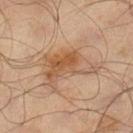Captured during whole-body skin photography for melanoma surveillance; the lesion was not biopsied. A roughly 15 mm field-of-view crop from a total-body skin photograph. The subject is a male approximately 65 years of age. The lesion is located on the leg. The tile uses cross-polarized illumination. Measured at roughly 7 mm in maximum diameter.